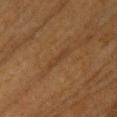follow-up = catalogued during a skin exam; not biopsied
size = ≈3.5 mm
site = the left upper arm
tile lighting = cross-polarized
patient = female, roughly 55 years of age
acquisition = total-body-photography crop, ~15 mm field of view